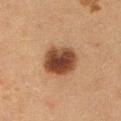A female patient about 50 years old. The lesion-visualizer software estimated border irregularity of about 1.5 on a 0–10 scale, a color-variation rating of about 5.5/10, and a peripheral color-asymmetry measure near 1.5. The lesion is located on the abdomen. This image is a 15 mm lesion crop taken from a total-body photograph. The lesion's longest dimension is about 4.5 mm.